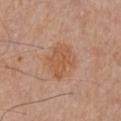Findings:
• biopsy status · imaged on a skin check; not biopsied
• lesion diameter · ≈4 mm
• tile lighting · white-light illumination
• patient · male, roughly 80 years of age
• image · ~15 mm crop, total-body skin-cancer survey
• anatomic site · the left upper arm